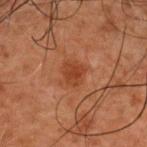Clinical impression: Imaged during a routine full-body skin examination; the lesion was not biopsied and no histopathology is available. Background: Captured under cross-polarized illumination. The lesion is located on the upper back. The lesion's longest dimension is about 3 mm. A male patient about 50 years old. Cropped from a whole-body photographic skin survey; the tile spans about 15 mm.